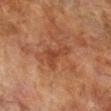The total-body-photography lesion software estimated an average lesion color of about L≈35 a*≈22 b*≈29 (CIELAB) and about 6 CIELAB-L* units darker than the surrounding skin. The analysis additionally found a border-irregularity index near 7.5/10, a within-lesion color-variation index near 1.5/10, and radial color variation of about 0. The software also gave a nevus-likeness score of about 0/100. The tile uses cross-polarized illumination. On the leg. A male subject aged 68–72. A 15 mm close-up extracted from a 3D total-body photography capture. The recorded lesion diameter is about 4 mm.